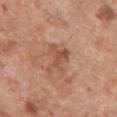Background: An algorithmic analysis of the crop reported border irregularity of about 6 on a 0–10 scale, a color-variation rating of about 3.5/10, and radial color variation of about 1.5. It also reported an automated nevus-likeness rating near 0 out of 100 and a detector confidence of about 100 out of 100 that the crop contains a lesion. A female subject aged approximately 50. Measured at roughly 4 mm in maximum diameter. A lesion tile, about 15 mm wide, cut from a 3D total-body photograph. Located on the chest. The tile uses white-light illumination.